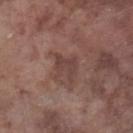Captured during whole-body skin photography for melanoma surveillance; the lesion was not biopsied. On the leg. The subject is a male in their mid- to late 70s. The recorded lesion diameter is about 3.5 mm. Cropped from a whole-body photographic skin survey; the tile spans about 15 mm. The tile uses white-light illumination.